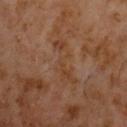illumination = cross-polarized
subject = male, aged 58 to 62
body site = the chest
size = ≈5.5 mm
acquisition = ~15 mm tile from a whole-body skin photo
automated lesion analysis = a lesion area of about 8.5 mm², an eccentricity of roughly 0.95, and a symmetry-axis asymmetry near 0.55; a border-irregularity index near 8.5/10 and internal color variation of about 2 on a 0–10 scale; a classifier nevus-likeness of about 0/100